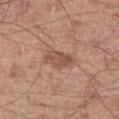notes=imaged on a skin check; not biopsied
subject=male, roughly 55 years of age
image=~15 mm crop, total-body skin-cancer survey
site=the left thigh
diameter=~4 mm (longest diameter)